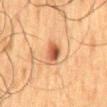biopsy status: catalogued during a skin exam; not biopsied | patient: male, aged around 60 | tile lighting: cross-polarized illumination | image source: 15 mm crop, total-body photography | diameter: about 3 mm | site: the mid back | automated metrics: an eccentricity of roughly 0.8.